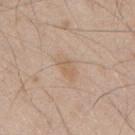The lesion was photographed on a routine skin check and not biopsied; there is no pathology result. Measured at roughly 2.5 mm in maximum diameter. The total-body-photography lesion software estimated a lesion color around L≈61 a*≈16 b*≈31 in CIELAB, about 6 CIELAB-L* units darker than the surrounding skin, and a lesion-to-skin contrast of about 5.5 (normalized; higher = more distinct). The software also gave a border-irregularity index near 2/10. A male subject aged 48–52. A region of skin cropped from a whole-body photographic capture, roughly 15 mm wide. This is a white-light tile. The lesion is located on the mid back.